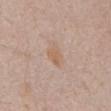Captured during whole-body skin photography for melanoma surveillance; the lesion was not biopsied.
The patient is a male in their mid- to late 60s.
The lesion is located on the abdomen.
A close-up tile cropped from a whole-body skin photograph, about 15 mm across.
The lesion-visualizer software estimated an area of roughly 4.5 mm², an eccentricity of roughly 0.7, and two-axis asymmetry of about 0.2. The software also gave a lesion-detection confidence of about 100/100.